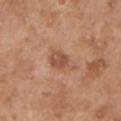<tbp_lesion>
  <image>
    <source>total-body photography crop</source>
    <field_of_view_mm>15</field_of_view_mm>
  </image>
  <patient>
    <sex>male</sex>
    <age_approx>55</age_approx>
  </patient>
  <lighting>white-light</lighting>
  <lesion_size>
    <long_diameter_mm_approx>3.5</long_diameter_mm_approx>
  </lesion_size>
  <site>left upper arm</site>
</tbp_lesion>